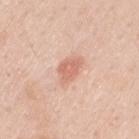Assessment: This lesion was catalogued during total-body skin photography and was not selected for biopsy. Image and clinical context: The lesion's longest dimension is about 3.5 mm. The lesion is on the left upper arm. The tile uses white-light illumination. An algorithmic analysis of the crop reported an outline eccentricity of about 0.75 (0 = round, 1 = elongated) and a shape-asymmetry score of about 0.3 (0 = symmetric). The analysis additionally found a border-irregularity rating of about 3/10 and peripheral color asymmetry of about 0.5. The software also gave an automated nevus-likeness rating near 90 out of 100 and lesion-presence confidence of about 100/100. A male patient, aged around 60. A close-up tile cropped from a whole-body skin photograph, about 15 mm across.